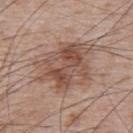Findings:
* follow-up · no biopsy performed (imaged during a skin exam)
* body site · the upper back
* imaging modality · 15 mm crop, total-body photography
* patient · male, aged 63 to 67